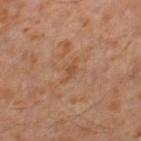Imaged during a routine full-body skin examination; the lesion was not biopsied and no histopathology is available.
The patient is a male about 30 years old.
About 3.5 mm across.
Located on the leg.
This is a cross-polarized tile.
A close-up tile cropped from a whole-body skin photograph, about 15 mm across.